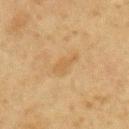– follow-up · no biopsy performed (imaged during a skin exam)
– image · 15 mm crop, total-body photography
– patient · male, aged approximately 65
– body site · the right upper arm